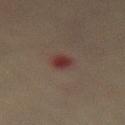{
  "lighting": "cross-polarized",
  "image": {
    "source": "total-body photography crop",
    "field_of_view_mm": 15
  },
  "site": "lower back",
  "patient": {
    "sex": "female",
    "age_approx": 80
  }
}